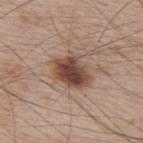<lesion>
<biopsy_status>not biopsied; imaged during a skin examination</biopsy_status>
<patient>
  <sex>male</sex>
  <age_approx>65</age_approx>
</patient>
<image>
  <source>total-body photography crop</source>
  <field_of_view_mm>15</field_of_view_mm>
</image>
<site>upper back</site>
</lesion>